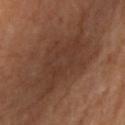workup — imaged on a skin check; not biopsied
image-analysis metrics — border irregularity of about 6 on a 0–10 scale, a within-lesion color-variation index near 3/10, and a peripheral color-asymmetry measure near 1
site — the right upper arm
imaging modality — 15 mm crop, total-body photography
lighting — cross-polarized
lesion diameter — about 9 mm
patient — female, roughly 70 years of age